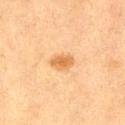No biopsy was performed on this lesion — it was imaged during a full skin examination and was not determined to be concerning. The tile uses cross-polarized illumination. The patient is a female in their 40s. Located on the right thigh. Automated image analysis of the tile measured a lesion color around L≈59 a*≈20 b*≈41 in CIELAB, a lesion–skin lightness drop of about 10, and a normalized border contrast of about 7. The software also gave border irregularity of about 1.5 on a 0–10 scale, internal color variation of about 2.5 on a 0–10 scale, and peripheral color asymmetry of about 0.5. It also reported a nevus-likeness score of about 85/100. A roughly 15 mm field-of-view crop from a total-body skin photograph.